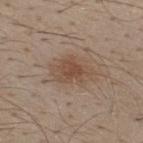{
  "biopsy_status": "not biopsied; imaged during a skin examination",
  "lighting": "white-light",
  "site": "back",
  "automated_metrics": {
    "area_mm2_approx": 9.5,
    "shape_asymmetry": 0.25,
    "border_irregularity_0_10": 4.0,
    "color_variation_0_10": 3.5,
    "peripheral_color_asymmetry": 1.0,
    "nevus_likeness_0_100": 90,
    "lesion_detection_confidence_0_100": 100
  },
  "patient": {
    "sex": "male",
    "age_approx": 30
  },
  "image": {
    "source": "total-body photography crop",
    "field_of_view_mm": 15
  },
  "lesion_size": {
    "long_diameter_mm_approx": 4.5
  }
}